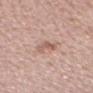| field | value |
|---|---|
| notes | imaged on a skin check; not biopsied |
| patient | male, about 45 years old |
| location | the head or neck |
| image | ~15 mm crop, total-body skin-cancer survey |
| lesion diameter | about 2.5 mm |
| tile lighting | white-light illumination |